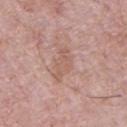| field | value |
|---|---|
| workup | imaged on a skin check; not biopsied |
| patient | male, approximately 55 years of age |
| size | ~4 mm (longest diameter) |
| lighting | white-light illumination |
| imaging modality | total-body-photography crop, ~15 mm field of view |
| TBP lesion metrics | an area of roughly 6.5 mm² and a symmetry-axis asymmetry near 0.35; a border-irregularity rating of about 4.5/10 and a color-variation rating of about 1.5/10; a classifier nevus-likeness of about 0/100 and a lesion-detection confidence of about 100/100 |